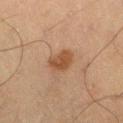biopsy status = no biopsy performed (imaged during a skin exam) | tile lighting = cross-polarized | acquisition = ~15 mm crop, total-body skin-cancer survey | location = the right thigh | patient = male, aged 68 to 72.